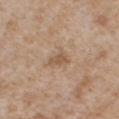Assessment:
Part of a total-body skin-imaging series; this lesion was reviewed on a skin check and was not flagged for biopsy.
Acquisition and patient details:
A male subject roughly 65 years of age. The tile uses white-light illumination. The lesion is located on the chest. An algorithmic analysis of the crop reported about 8 CIELAB-L* units darker than the surrounding skin and a normalized border contrast of about 6. The analysis additionally found a within-lesion color-variation index near 1.5/10 and peripheral color asymmetry of about 0.5. And it measured a classifier nevus-likeness of about 0/100 and lesion-presence confidence of about 100/100. Measured at roughly 2.5 mm in maximum diameter. A close-up tile cropped from a whole-body skin photograph, about 15 mm across.